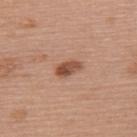Recorded during total-body skin imaging; not selected for excision or biopsy. A female patient, in their 60s. The tile uses white-light illumination. The lesion is located on the upper back. An algorithmic analysis of the crop reported a border-irregularity index near 2/10, a color-variation rating of about 5/10, and radial color variation of about 2. This image is a 15 mm lesion crop taken from a total-body photograph. Approximately 3 mm at its widest.